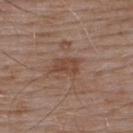The lesion was photographed on a routine skin check and not biopsied; there is no pathology result. An algorithmic analysis of the crop reported a lesion color around L≈45 a*≈20 b*≈27 in CIELAB and a lesion–skin lightness drop of about 7. It also reported border irregularity of about 3 on a 0–10 scale, internal color variation of about 2.5 on a 0–10 scale, and radial color variation of about 0.5. The analysis additionally found an automated nevus-likeness rating near 15 out of 100 and a detector confidence of about 100 out of 100 that the crop contains a lesion. Imaged with white-light lighting. Measured at roughly 3.5 mm in maximum diameter. The subject is a male roughly 55 years of age. The lesion is on the upper back. A roughly 15 mm field-of-view crop from a total-body skin photograph.